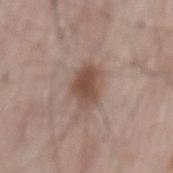workup: catalogued during a skin exam; not biopsied
image: ~15 mm crop, total-body skin-cancer survey
illumination: white-light illumination
subject: male, in their mid- to late 60s
image-analysis metrics: a footprint of about 8 mm², an outline eccentricity of about 0.8 (0 = round, 1 = elongated), and two-axis asymmetry of about 0.25; a border-irregularity index near 2.5/10 and a within-lesion color-variation index near 3.5/10; a classifier nevus-likeness of about 95/100 and a detector confidence of about 100 out of 100 that the crop contains a lesion
lesion diameter: about 4 mm
anatomic site: the back